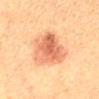Q: Was this lesion biopsied?
A: no biopsy performed (imaged during a skin exam)
Q: Automated lesion metrics?
A: border irregularity of about 3 on a 0–10 scale and radial color variation of about 2.5; an automated nevus-likeness rating near 95 out of 100 and a detector confidence of about 100 out of 100 that the crop contains a lesion
Q: Lesion location?
A: the mid back
Q: Illumination type?
A: cross-polarized illumination
Q: What kind of image is this?
A: total-body-photography crop, ~15 mm field of view
Q: Patient demographics?
A: male, aged around 50
Q: Lesion size?
A: about 7 mm